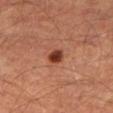{
  "biopsy_status": "not biopsied; imaged during a skin examination",
  "image": {
    "source": "total-body photography crop",
    "field_of_view_mm": 15
  },
  "patient": {
    "sex": "male",
    "age_approx": 65
  },
  "lesion_size": {
    "long_diameter_mm_approx": 2.5
  },
  "automated_metrics": {
    "area_mm2_approx": 3.5,
    "shape_asymmetry": 0.2,
    "border_irregularity_0_10": 1.5,
    "color_variation_0_10": 2.0,
    "peripheral_color_asymmetry": 0.5
  },
  "site": "right thigh",
  "lighting": "cross-polarized"
}